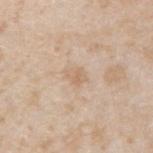<record>
  <biopsy_status>not biopsied; imaged during a skin examination</biopsy_status>
  <lighting>white-light</lighting>
  <lesion_size>
    <long_diameter_mm_approx>2.5</long_diameter_mm_approx>
  </lesion_size>
  <site>right forearm</site>
  <patient>
    <sex>male</sex>
    <age_approx>45</age_approx>
  </patient>
  <image>
    <source>total-body photography crop</source>
    <field_of_view_mm>15</field_of_view_mm>
  </image>
  <automated_metrics>
    <area_mm2_approx>3.5</area_mm2_approx>
    <eccentricity>0.65</eccentricity>
    <cielab_L>65</cielab_L>
    <cielab_a>15</cielab_a>
    <cielab_b>32</cielab_b>
    <vs_skin_contrast_norm>5.0</vs_skin_contrast_norm>
    <nevus_likeness_0_100>0</nevus_likeness_0_100>
    <lesion_detection_confidence_0_100>100</lesion_detection_confidence_0_100>
  </automated_metrics>
</record>